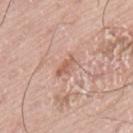This lesion was catalogued during total-body skin photography and was not selected for biopsy. A region of skin cropped from a whole-body photographic capture, roughly 15 mm wide. The lesion-visualizer software estimated a lesion area of about 3.5 mm², an outline eccentricity of about 0.9 (0 = round, 1 = elongated), and a symmetry-axis asymmetry near 0.25. It also reported border irregularity of about 3 on a 0–10 scale, a color-variation rating of about 1/10, and radial color variation of about 0. And it measured an automated nevus-likeness rating near 0 out of 100 and a detector confidence of about 100 out of 100 that the crop contains a lesion. The recorded lesion diameter is about 3 mm. Captured under white-light illumination. Located on the back. The patient is a male aged 73 to 77.